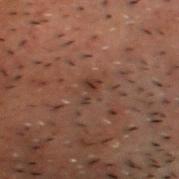No biopsy was performed on this lesion — it was imaged during a full skin examination and was not determined to be concerning. A male patient in their 50s. Captured under cross-polarized illumination. From the chest. This image is a 15 mm lesion crop taken from a total-body photograph. Measured at roughly 2.5 mm in maximum diameter. The lesion-visualizer software estimated an area of roughly 3 mm², an outline eccentricity of about 0.8 (0 = round, 1 = elongated), and a shape-asymmetry score of about 0.55 (0 = symmetric). And it measured a mean CIELAB color near L≈26 a*≈15 b*≈20, a lesion–skin lightness drop of about 6, and a normalized border contrast of about 6.5. The software also gave a lesion-detection confidence of about 95/100.